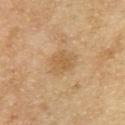Notes:
– biopsy status: total-body-photography surveillance lesion; no biopsy
– image: ~15 mm crop, total-body skin-cancer survey
– patient: female, aged around 60
– automated lesion analysis: a classifier nevus-likeness of about 5/100 and a detector confidence of about 100 out of 100 that the crop contains a lesion
– location: the chest
– lighting: cross-polarized
– diameter: about 2.5 mm